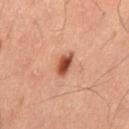Clinical impression: Captured during whole-body skin photography for melanoma surveillance; the lesion was not biopsied. Clinical summary: A 15 mm close-up tile from a total-body photography series done for melanoma screening. The patient is a male aged around 60. Imaged with cross-polarized lighting. Located on the mid back. An algorithmic analysis of the crop reported a lesion color around L≈37 a*≈23 b*≈27 in CIELAB, about 13 CIELAB-L* units darker than the surrounding skin, and a lesion-to-skin contrast of about 11 (normalized; higher = more distinct).